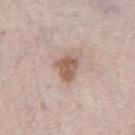<lesion>
<biopsy_status>not biopsied; imaged during a skin examination</biopsy_status>
<image>
  <source>total-body photography crop</source>
  <field_of_view_mm>15</field_of_view_mm>
</image>
<lighting>white-light</lighting>
<patient>
  <sex>male</sex>
  <age_approx>75</age_approx>
</patient>
<lesion_size>
  <long_diameter_mm_approx>3.0</long_diameter_mm_approx>
</lesion_size>
<site>chest</site>
</lesion>